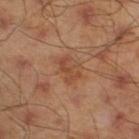Part of a total-body skin-imaging series; this lesion was reviewed on a skin check and was not flagged for biopsy. Located on the left lower leg. The tile uses cross-polarized illumination. A male subject in their mid- to late 40s. The total-body-photography lesion software estimated a lesion area of about 6.5 mm² and a shape-asymmetry score of about 0.25 (0 = symmetric). Cropped from a whole-body photographic skin survey; the tile spans about 15 mm. Approximately 3.5 mm at its widest.